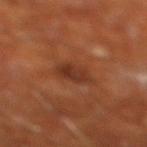Findings:
* notes · imaged on a skin check; not biopsied
* location · the right lower leg
* illumination · cross-polarized illumination
* image · 15 mm crop, total-body photography
* patient · male, about 65 years old